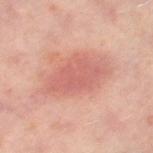No biopsy was performed on this lesion — it was imaged during a full skin examination and was not determined to be concerning.
Captured under cross-polarized illumination.
Measured at roughly 7 mm in maximum diameter.
A female subject in their 50s.
Cropped from a total-body skin-imaging series; the visible field is about 15 mm.
The lesion is on the left thigh.